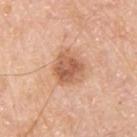workup: catalogued during a skin exam; not biopsied | body site: the upper back | diameter: about 4 mm | image source: ~15 mm crop, total-body skin-cancer survey | TBP lesion metrics: an area of roughly 10 mm², a shape eccentricity near 0.5, and a shape-asymmetry score of about 0.2 (0 = symmetric); a border-irregularity rating of about 2/10, a color-variation rating of about 5/10, and peripheral color asymmetry of about 2; a nevus-likeness score of about 35/100 and lesion-presence confidence of about 100/100 | patient: male, in their 80s | illumination: white-light.